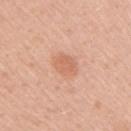| feature | finding |
|---|---|
| biopsy status | catalogued during a skin exam; not biopsied |
| site | the arm |
| illumination | white-light illumination |
| patient | female, aged approximately 30 |
| lesion size | about 2.5 mm |
| acquisition | ~15 mm crop, total-body skin-cancer survey |
| automated lesion analysis | border irregularity of about 2 on a 0–10 scale and radial color variation of about 0.5; a classifier nevus-likeness of about 15/100 |